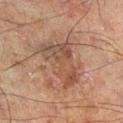follow-up = imaged on a skin check; not biopsied | lesion size = about 6 mm | body site = the left leg | lighting = cross-polarized | subject = male, aged 58 to 62 | image source = ~15 mm tile from a whole-body skin photo.